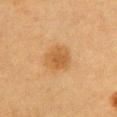This lesion was catalogued during total-body skin photography and was not selected for biopsy. A 15 mm close-up extracted from a 3D total-body photography capture. From the left upper arm. The subject is a female in their 40s. Longest diameter approximately 3.5 mm. The lesion-visualizer software estimated a lesion area of about 9.5 mm² and a symmetry-axis asymmetry near 0.15. The software also gave a mean CIELAB color near L≈47 a*≈18 b*≈36, roughly 7 lightness units darker than nearby skin, and a normalized lesion–skin contrast near 6.5. It also reported a color-variation rating of about 2/10 and a peripheral color-asymmetry measure near 0.5.